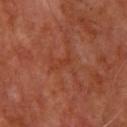Q: Was a biopsy performed?
A: total-body-photography surveillance lesion; no biopsy